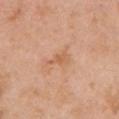The lesion was photographed on a routine skin check and not biopsied; there is no pathology result.
The tile uses white-light illumination.
Automated image analysis of the tile measured a lesion area of about 2.5 mm², a shape eccentricity near 0.85, and two-axis asymmetry of about 0.5. The analysis additionally found a classifier nevus-likeness of about 0/100.
A 15 mm close-up extracted from a 3D total-body photography capture.
On the arm.
A female subject, approximately 40 years of age.